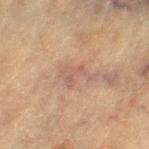Notes:
– workup · imaged on a skin check; not biopsied
– size · about 4 mm
– location · the left thigh
– patient · female, roughly 80 years of age
– illumination · cross-polarized illumination
– TBP lesion metrics · a border-irregularity index near 7.5/10, a color-variation rating of about 2.5/10, and peripheral color asymmetry of about 0.5; a lesion-detection confidence of about 95/100
– image source · ~15 mm crop, total-body skin-cancer survey